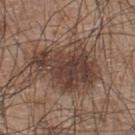image:
  source: total-body photography crop
  field_of_view_mm: 15
lesion_size:
  long_diameter_mm_approx: 7.5
site: upper back
automated_metrics:
  area_mm2_approx: 28.0
  eccentricity: 0.75
  cielab_L: 40
  cielab_a: 17
  cielab_b: 23
  vs_skin_darker_L: 11.0
  border_irregularity_0_10: 5.0
  color_variation_0_10: 5.0
  peripheral_color_asymmetry: 2.0
  nevus_likeness_0_100: 40
patient:
  sex: male
  age_approx: 45